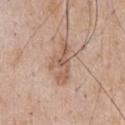Part of a total-body skin-imaging series; this lesion was reviewed on a skin check and was not flagged for biopsy.
On the chest.
Imaged with white-light lighting.
A male subject aged around 60.
Cropped from a whole-body photographic skin survey; the tile spans about 15 mm.
An algorithmic analysis of the crop reported a mean CIELAB color near L≈58 a*≈19 b*≈29, a lesion–skin lightness drop of about 10, and a normalized border contrast of about 7. And it measured a border-irregularity rating of about 7.5/10 and a color-variation rating of about 3.5/10. It also reported an automated nevus-likeness rating near 10 out of 100 and a detector confidence of about 100 out of 100 that the crop contains a lesion.